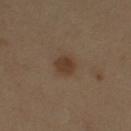Q: Was a biopsy performed?
A: no biopsy performed (imaged during a skin exam)